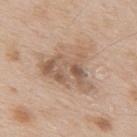Q: Was a biopsy performed?
A: catalogued during a skin exam; not biopsied
Q: How was the tile lit?
A: white-light
Q: How was this image acquired?
A: total-body-photography crop, ~15 mm field of view
Q: Lesion location?
A: the upper back
Q: Who is the patient?
A: male, roughly 65 years of age
Q: How large is the lesion?
A: ~7 mm (longest diameter)
Q: What did automated image analysis measure?
A: a lesion area of about 21 mm², an outline eccentricity of about 0.6 (0 = round, 1 = elongated), and two-axis asymmetry of about 0.45; an average lesion color of about L≈58 a*≈16 b*≈29 (CIELAB), a lesion–skin lightness drop of about 10, and a normalized border contrast of about 6.5; a classifier nevus-likeness of about 10/100 and a lesion-detection confidence of about 100/100